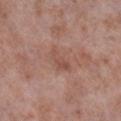Case summary:
• notes — imaged on a skin check; not biopsied
• image source — ~15 mm tile from a whole-body skin photo
• location — the leg
• subject — male, aged 53 to 57
• automated lesion analysis — about 7 CIELAB-L* units darker than the surrounding skin and a lesion-to-skin contrast of about 5 (normalized; higher = more distinct); a border-irregularity rating of about 4.5/10, a color-variation rating of about 1/10, and a peripheral color-asymmetry measure near 0.5; a classifier nevus-likeness of about 0/100 and a lesion-detection confidence of about 100/100